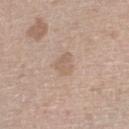Captured during whole-body skin photography for melanoma surveillance; the lesion was not biopsied.
A 15 mm close-up tile from a total-body photography series done for melanoma screening.
Automated tile analysis of the lesion measured a color-variation rating of about 1.5/10 and peripheral color asymmetry of about 0.5.
Captured under white-light illumination.
The lesion is on the right lower leg.
A female subject aged approximately 70.
The recorded lesion diameter is about 2.5 mm.